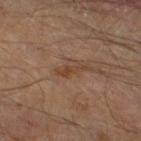Q: Is there a histopathology result?
A: imaged on a skin check; not biopsied
Q: Patient demographics?
A: male, in their 70s
Q: How was the tile lit?
A: cross-polarized illumination
Q: Where on the body is the lesion?
A: the right thigh
Q: How was this image acquired?
A: 15 mm crop, total-body photography